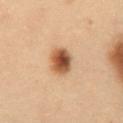Q: Patient demographics?
A: male, roughly 55 years of age
Q: What did automated image analysis measure?
A: roughly 15 lightness units darker than nearby skin and a normalized lesion–skin contrast near 11.5; border irregularity of about 1.5 on a 0–10 scale, internal color variation of about 6.5 on a 0–10 scale, and a peripheral color-asymmetry measure near 2; an automated nevus-likeness rating near 100 out of 100 and a detector confidence of about 100 out of 100 that the crop contains a lesion
Q: What is the anatomic site?
A: the abdomen
Q: What kind of image is this?
A: 15 mm crop, total-body photography